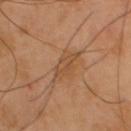{
  "biopsy_status": "not biopsied; imaged during a skin examination",
  "image": {
    "source": "total-body photography crop",
    "field_of_view_mm": 15
  },
  "lesion_size": {
    "long_diameter_mm_approx": 4.5
  },
  "site": "upper back",
  "patient": {
    "sex": "male",
    "age_approx": 40
  }
}